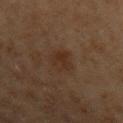follow-up = imaged on a skin check; not biopsied | diameter = about 3.5 mm | subject = male, in their 70s | site = the left upper arm | tile lighting = cross-polarized | imaging modality = ~15 mm crop, total-body skin-cancer survey.